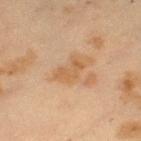Notes:
- notes · imaged on a skin check; not biopsied
- patient · female, aged 38 to 42
- TBP lesion metrics · a footprint of about 6 mm² and a shape eccentricity near 0.85; border irregularity of about 5 on a 0–10 scale, a color-variation rating of about 2/10, and peripheral color asymmetry of about 0.5
- site · the left thigh
- tile lighting · cross-polarized illumination
- size · ~4 mm (longest diameter)
- image · total-body-photography crop, ~15 mm field of view